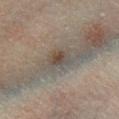Impression:
Imaged during a routine full-body skin examination; the lesion was not biopsied and no histopathology is available.
Image and clinical context:
A 15 mm close-up extracted from a 3D total-body photography capture. Imaged with cross-polarized lighting. The subject is a male aged 43 to 47. Approximately 2.5 mm at its widest. The total-body-photography lesion software estimated a footprint of about 4.5 mm² and an eccentricity of roughly 0.55. The software also gave a mean CIELAB color near L≈38 a*≈8 b*≈18, a lesion–skin lightness drop of about 7, and a lesion-to-skin contrast of about 7 (normalized; higher = more distinct). And it measured border irregularity of about 3 on a 0–10 scale, internal color variation of about 5 on a 0–10 scale, and peripheral color asymmetry of about 1.5. The software also gave an automated nevus-likeness rating near 75 out of 100 and a lesion-detection confidence of about 85/100. From the right lower leg.